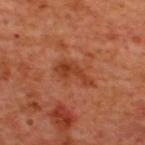<record>
<lesion_size>
  <long_diameter_mm_approx>4.5</long_diameter_mm_approx>
</lesion_size>
<patient>
  <sex>male</sex>
  <age_approx>50</age_approx>
</patient>
<site>upper back</site>
<image>
  <source>total-body photography crop</source>
  <field_of_view_mm>15</field_of_view_mm>
</image>
<lighting>cross-polarized</lighting>
</record>